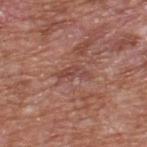Part of a total-body skin-imaging series; this lesion was reviewed on a skin check and was not flagged for biopsy.
The recorded lesion diameter is about 3.5 mm.
Captured under white-light illumination.
A male subject, aged around 65.
The lesion is located on the upper back.
A 15 mm crop from a total-body photograph taken for skin-cancer surveillance.